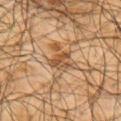Clinical impression: The lesion was tiled from a total-body skin photograph and was not biopsied. Image and clinical context: An algorithmic analysis of the crop reported about 11 CIELAB-L* units darker than the surrounding skin and a lesion-to-skin contrast of about 8 (normalized; higher = more distinct). And it measured a nevus-likeness score of about 70/100 and a detector confidence of about 80 out of 100 that the crop contains a lesion. Approximately 3 mm at its widest. A male patient, in their mid-60s. Located on the upper back. This is a cross-polarized tile. A lesion tile, about 15 mm wide, cut from a 3D total-body photograph.